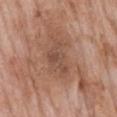No biopsy was performed on this lesion — it was imaged during a full skin examination and was not determined to be concerning.
Located on the back.
A region of skin cropped from a whole-body photographic capture, roughly 15 mm wide.
A male patient, roughly 75 years of age.